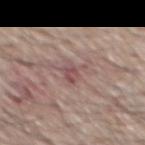Recorded during total-body skin imaging; not selected for excision or biopsy.
A male subject aged 63 to 67.
The recorded lesion diameter is about 3 mm.
Automated image analysis of the tile measured a border-irregularity rating of about 6/10, a within-lesion color-variation index near 2.5/10, and a peripheral color-asymmetry measure near 0.5.
The tile uses white-light illumination.
Located on the mid back.
A region of skin cropped from a whole-body photographic capture, roughly 15 mm wide.